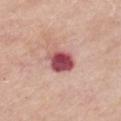Part of a total-body skin-imaging series; this lesion was reviewed on a skin check and was not flagged for biopsy. Captured under white-light illumination. A female subject aged 68 to 72. A region of skin cropped from a whole-body photographic capture, roughly 15 mm wide. Automated tile analysis of the lesion measured an outline eccentricity of about 0.5 (0 = round, 1 = elongated) and a shape-asymmetry score of about 0.2 (0 = symmetric). And it measured a color-variation rating of about 7/10 and radial color variation of about 2. The software also gave a classifier nevus-likeness of about 0/100. Located on the chest.